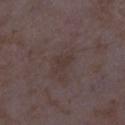| key | value |
|---|---|
| biopsy status | imaged on a skin check; not biopsied |
| location | the right lower leg |
| subject | female, approximately 35 years of age |
| size | ≈2.5 mm |
| lighting | white-light |
| image source | total-body-photography crop, ~15 mm field of view |